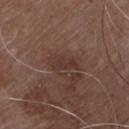<record>
  <patient>
    <sex>male</sex>
    <age_approx>80</age_approx>
  </patient>
  <site>front of the torso</site>
  <image>
    <source>total-body photography crop</source>
    <field_of_view_mm>15</field_of_view_mm>
  </image>
</record>